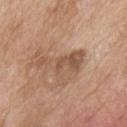– notes — no biopsy performed (imaged during a skin exam)
– image — ~15 mm crop, total-body skin-cancer survey
– lesion size — ~6.5 mm (longest diameter)
– site — the back
– subject — male, roughly 80 years of age
– illumination — white-light